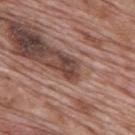biopsy status: imaged on a skin check; not biopsied
subject: male, aged 68 to 72
illumination: white-light
site: the mid back
TBP lesion metrics: a lesion area of about 3.5 mm², an outline eccentricity of about 0.85 (0 = round, 1 = elongated), and two-axis asymmetry of about 0.35; a mean CIELAB color near L≈41 a*≈20 b*≈22, a lesion–skin lightness drop of about 12, and a normalized border contrast of about 10; a border-irregularity index near 4/10, internal color variation of about 2.5 on a 0–10 scale, and a peripheral color-asymmetry measure near 0.5
image source: ~15 mm crop, total-body skin-cancer survey
lesion diameter: about 3 mm